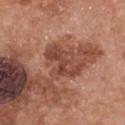biopsy_status: not biopsied; imaged during a skin examination